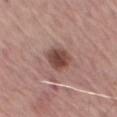Q: Is there a histopathology result?
A: catalogued during a skin exam; not biopsied
Q: How was this image acquired?
A: ~15 mm tile from a whole-body skin photo
Q: Lesion size?
A: ≈3 mm
Q: How was the tile lit?
A: white-light illumination
Q: What is the anatomic site?
A: the left thigh
Q: Who is the patient?
A: male, aged 73 to 77